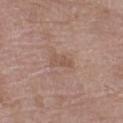Q: Was a biopsy performed?
A: imaged on a skin check; not biopsied
Q: Who is the patient?
A: female, approximately 70 years of age
Q: How was this image acquired?
A: ~15 mm tile from a whole-body skin photo
Q: Lesion location?
A: the left thigh